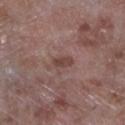Imaged during a routine full-body skin examination; the lesion was not biopsied and no histopathology is available.
On the left lower leg.
The patient is a male aged 53–57.
The lesion's longest dimension is about 2.5 mm.
Cropped from a whole-body photographic skin survey; the tile spans about 15 mm.